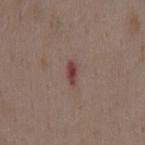biopsy status = no biopsy performed (imaged during a skin exam)
image-analysis metrics = an area of roughly 3 mm², a shape eccentricity near 0.85, and two-axis asymmetry of about 0.35; a mean CIELAB color near L≈42 a*≈22 b*≈19 and roughly 10 lightness units darker than nearby skin; border irregularity of about 3 on a 0–10 scale and a peripheral color-asymmetry measure near 0.5
site = the mid back
patient = female, aged 33–37
illumination = white-light illumination
imaging modality = 15 mm crop, total-body photography
diameter = ≈2.5 mm